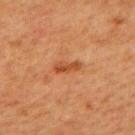  image:
    source: total-body photography crop
    field_of_view_mm: 15
  automated_metrics:
    area_mm2_approx: 3.5
    eccentricity: 0.9
    border_irregularity_0_10: 3.5
    color_variation_0_10: 1.5
    peripheral_color_asymmetry: 0.5
    lesion_detection_confidence_0_100: 100
  lesion_size:
    long_diameter_mm_approx: 3.0
  site: back
  patient:
    sex: female
    age_approx: 40
  lighting: cross-polarized